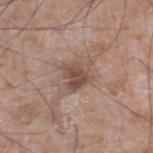automated metrics — a footprint of about 6 mm² and a symmetry-axis asymmetry near 0.3; border irregularity of about 3 on a 0–10 scale, internal color variation of about 3.5 on a 0–10 scale, and peripheral color asymmetry of about 1.5; an automated nevus-likeness rating near 20 out of 100 and a lesion-detection confidence of about 100/100 | patient — male, approximately 60 years of age | lesion size — ≈3 mm | anatomic site — the leg | acquisition — ~15 mm crop, total-body skin-cancer survey.